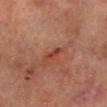* follow-up — imaged on a skin check; not biopsied
* size — ≈3 mm
* tile lighting — cross-polarized
* anatomic site — the left lower leg
* patient — male, aged approximately 70
* image — ~15 mm tile from a whole-body skin photo